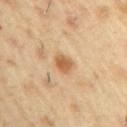{"biopsy_status": "not biopsied; imaged during a skin examination", "image": {"source": "total-body photography crop", "field_of_view_mm": 15}, "lesion_size": {"long_diameter_mm_approx": 2.5}, "patient": {"sex": "male", "age_approx": 55}, "site": "right upper arm"}